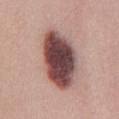biopsy status = total-body-photography surveillance lesion; no biopsy
location = the chest
lighting = white-light
lesion diameter = ≈8.5 mm
patient = female, approximately 40 years of age
TBP lesion metrics = a lesion–skin lightness drop of about 22 and a normalized lesion–skin contrast near 15; a lesion-detection confidence of about 100/100
image = ~15 mm crop, total-body skin-cancer survey